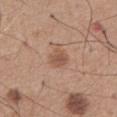{"biopsy_status": "not biopsied; imaged during a skin examination"}